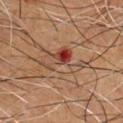Background:
Automated image analysis of the tile measured a lesion–skin lightness drop of about 7 and a normalized lesion–skin contrast near 6.5. And it measured a within-lesion color-variation index near 10/10 and peripheral color asymmetry of about 6. A 15 mm close-up tile from a total-body photography series done for melanoma screening. A male subject, aged around 65. Captured under cross-polarized illumination. The lesion is located on the front of the torso.